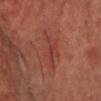<case>
<lighting>cross-polarized</lighting>
<patient>
  <sex>male</sex>
  <age_approx>70</age_approx>
</patient>
<lesion_size>
  <long_diameter_mm_approx>4.5</long_diameter_mm_approx>
</lesion_size>
<image>
  <source>total-body photography crop</source>
  <field_of_view_mm>15</field_of_view_mm>
</image>
<site>chest</site>
</case>